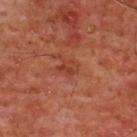Part of a total-body skin-imaging series; this lesion was reviewed on a skin check and was not flagged for biopsy. Cropped from a whole-body photographic skin survey; the tile spans about 15 mm. A male subject, aged around 60. On the front of the torso.